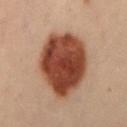{
  "biopsy_status": "not biopsied; imaged during a skin examination",
  "site": "lower back",
  "image": {
    "source": "total-body photography crop",
    "field_of_view_mm": 15
  },
  "patient": {
    "sex": "female",
    "age_approx": 30
  }
}